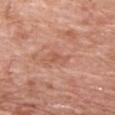biopsy status: imaged on a skin check; not biopsied
body site: the chest
lighting: white-light
imaging modality: ~15 mm tile from a whole-body skin photo
diameter: about 2.5 mm
patient: male, about 60 years old
image-analysis metrics: a lesion area of about 2.5 mm² and a symmetry-axis asymmetry near 0.6; roughly 7 lightness units darker than nearby skin and a lesion-to-skin contrast of about 5 (normalized; higher = more distinct); a classifier nevus-likeness of about 0/100 and lesion-presence confidence of about 100/100